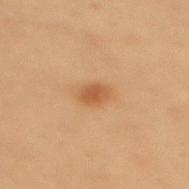| field | value |
|---|---|
| follow-up | total-body-photography surveillance lesion; no biopsy |
| patient | female, aged 38 to 42 |
| site | the upper back |
| tile lighting | cross-polarized illumination |
| automated metrics | a lesion area of about 4 mm² and a symmetry-axis asymmetry near 0.25; a mean CIELAB color near L≈45 a*≈19 b*≈33, a lesion–skin lightness drop of about 9, and a normalized border contrast of about 7; border irregularity of about 2.5 on a 0–10 scale, a within-lesion color-variation index near 1.5/10, and a peripheral color-asymmetry measure near 0.5; a classifier nevus-likeness of about 90/100 and a detector confidence of about 100 out of 100 that the crop contains a lesion |
| image source | ~15 mm tile from a whole-body skin photo |
| lesion size | about 3 mm |